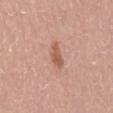Clinical summary:
The lesion is located on the mid back. A close-up tile cropped from a whole-body skin photograph, about 15 mm across. The recorded lesion diameter is about 3 mm. A female subject, roughly 65 years of age.